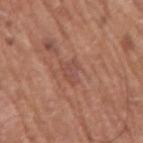Imaged during a routine full-body skin examination; the lesion was not biopsied and no histopathology is available.